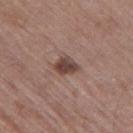Captured during whole-body skin photography for melanoma surveillance; the lesion was not biopsied. On the leg. A male patient aged around 65. The lesion's longest dimension is about 2.5 mm. A roughly 15 mm field-of-view crop from a total-body skin photograph. An algorithmic analysis of the crop reported a lesion area of about 5 mm², an outline eccentricity of about 0.6 (0 = round, 1 = elongated), and a symmetry-axis asymmetry near 0.25.